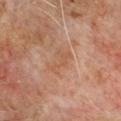The lesion was tiled from a total-body skin photograph and was not biopsied.
On the chest.
Imaged with cross-polarized lighting.
A male patient aged 63–67.
Cropped from a whole-body photographic skin survey; the tile spans about 15 mm.
Approximately 3 mm at its widest.